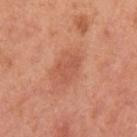Acquisition and patient details: Automated image analysis of the tile measured an area of roughly 4 mm², an outline eccentricity of about 0.8 (0 = round, 1 = elongated), and a shape-asymmetry score of about 0.5 (0 = symmetric). And it measured a lesion color around L≈55 a*≈29 b*≈34 in CIELAB and about 7 CIELAB-L* units darker than the surrounding skin. About 3.5 mm across. On the arm. A female patient, about 40 years old. The tile uses white-light illumination. A 15 mm close-up tile from a total-body photography series done for melanoma screening.